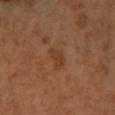body site: the leg | subject: female, about 65 years old | imaging modality: 15 mm crop, total-body photography.